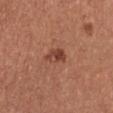Imaged during a routine full-body skin examination; the lesion was not biopsied and no histopathology is available.
A female patient in their mid-40s.
The tile uses white-light illumination.
A roughly 15 mm field-of-view crop from a total-body skin photograph.
The lesion is on the chest.
Measured at roughly 3 mm in maximum diameter.